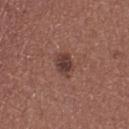biopsy_status: not biopsied; imaged during a skin examination
lesion_size:
  long_diameter_mm_approx: 3.0
site: upper back
lighting: white-light
patient:
  sex: female
  age_approx: 25
image:
  source: total-body photography crop
  field_of_view_mm: 15